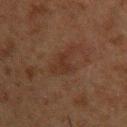– workup — total-body-photography surveillance lesion; no biopsy
– tile lighting — cross-polarized illumination
– patient — male, in their 50s
– image source — 15 mm crop, total-body photography
– body site — the left upper arm
– automated metrics — a shape eccentricity near 0.85; about 5 CIELAB-L* units darker than the surrounding skin; a classifier nevus-likeness of about 5/100 and a detector confidence of about 100 out of 100 that the crop contains a lesion
– diameter — ~5 mm (longest diameter)